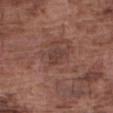Q: Is there a histopathology result?
A: imaged on a skin check; not biopsied
Q: What kind of image is this?
A: 15 mm crop, total-body photography
Q: What is the lesion's diameter?
A: about 2.5 mm
Q: Where on the body is the lesion?
A: the right forearm
Q: Illumination type?
A: white-light illumination
Q: Who is the patient?
A: male, in their mid-70s